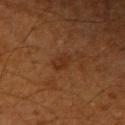Captured under cross-polarized illumination.
A male patient aged around 60.
Located on the arm.
The recorded lesion diameter is about 2.5 mm.
A 15 mm close-up tile from a total-body photography series done for melanoma screening.